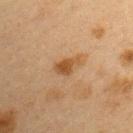Assessment:
This lesion was catalogued during total-body skin photography and was not selected for biopsy.
Clinical summary:
The lesion's longest dimension is about 3 mm. The lesion-visualizer software estimated a footprint of about 4 mm², a shape eccentricity near 0.8, and a shape-asymmetry score of about 0.35 (0 = symmetric). It also reported a lesion color around L≈41 a*≈18 b*≈33 in CIELAB. And it measured a border-irregularity index near 3.5/10 and radial color variation of about 0.5. A female patient, aged approximately 40. The lesion is on the left upper arm. Cropped from a whole-body photographic skin survey; the tile spans about 15 mm.